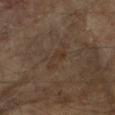Recorded during total-body skin imaging; not selected for excision or biopsy. A male patient aged 63 to 67. Automated image analysis of the tile measured a mean CIELAB color near L≈33 a*≈13 b*≈24 and about 4 CIELAB-L* units darker than the surrounding skin. And it measured a border-irregularity index near 5/10, a within-lesion color-variation index near 2.5/10, and peripheral color asymmetry of about 0.5. From the right forearm. This is a cross-polarized tile. About 4 mm across. Cropped from a total-body skin-imaging series; the visible field is about 15 mm.